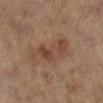{
  "biopsy_status": "not biopsied; imaged during a skin examination",
  "patient": {
    "sex": "female",
    "age_approx": 60
  },
  "image": {
    "source": "total-body photography crop",
    "field_of_view_mm": 15
  },
  "site": "left lower leg"
}